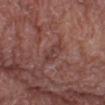The lesion was tiled from a total-body skin photograph and was not biopsied.
A lesion tile, about 15 mm wide, cut from a 3D total-body photograph.
A male subject, about 80 years old.
From the left lower leg.